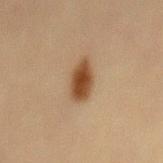Assessment:
Imaged during a routine full-body skin examination; the lesion was not biopsied and no histopathology is available.
Clinical summary:
The recorded lesion diameter is about 4.5 mm. On the mid back. A 15 mm crop from a total-body photograph taken for skin-cancer surveillance. The subject is a male aged 58 to 62. The tile uses cross-polarized illumination.